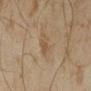Imaged during a routine full-body skin examination; the lesion was not biopsied and no histopathology is available. The lesion-visualizer software estimated a border-irregularity index near 3.5/10 and a color-variation rating of about 1/10. Measured at roughly 2.5 mm in maximum diameter. A 15 mm crop from a total-body photograph taken for skin-cancer surveillance. A male subject aged 43–47. On the left forearm. Imaged with cross-polarized lighting.